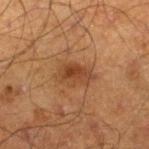Findings:
* biopsy status: no biopsy performed (imaged during a skin exam)